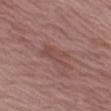biopsy_status: not biopsied; imaged during a skin examination
image:
  source: total-body photography crop
  field_of_view_mm: 15
lesion_size:
  long_diameter_mm_approx: 4.5
site: left thigh
patient:
  sex: female
  age_approx: 65
automated_metrics:
  area_mm2_approx: 7.0
  eccentricity: 0.9